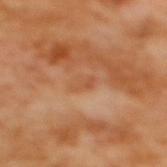Part of a total-body skin-imaging series; this lesion was reviewed on a skin check and was not flagged for biopsy. Located on the upper back. A female patient, aged approximately 55. Cropped from a whole-body photographic skin survey; the tile spans about 15 mm. The total-body-photography lesion software estimated an area of roughly 3 mm², a shape eccentricity near 0.85, and a symmetry-axis asymmetry near 0.35. The analysis additionally found a normalized lesion–skin contrast near 5.5. The analysis additionally found a border-irregularity index near 3/10 and peripheral color asymmetry of about 1. The tile uses cross-polarized illumination.